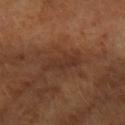Captured during whole-body skin photography for melanoma surveillance; the lesion was not biopsied. This is a cross-polarized tile. On the right forearm. Cropped from a total-body skin-imaging series; the visible field is about 15 mm. The total-body-photography lesion software estimated an area of roughly 9.5 mm² and two-axis asymmetry of about 0.35. The software also gave a lesion–skin lightness drop of about 6 and a lesion-to-skin contrast of about 6 (normalized; higher = more distinct). It also reported a border-irregularity rating of about 4.5/10. The software also gave a nevus-likeness score of about 0/100 and lesion-presence confidence of about 85/100. A female patient. Longest diameter approximately 5 mm.